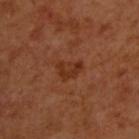Q: Was this lesion biopsied?
A: no biopsy performed (imaged during a skin exam)
Q: What are the patient's age and sex?
A: male, aged 48 to 52
Q: Lesion size?
A: ≈3 mm
Q: Lesion location?
A: the upper back
Q: What kind of image is this?
A: ~15 mm tile from a whole-body skin photo